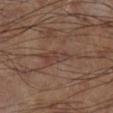Clinical impression: The lesion was photographed on a routine skin check and not biopsied; there is no pathology result. Image and clinical context: A region of skin cropped from a whole-body photographic capture, roughly 15 mm wide. About 3.5 mm across. Located on the right lower leg. An algorithmic analysis of the crop reported a border-irregularity index near 4/10 and a within-lesion color-variation index near 2.5/10. This is a cross-polarized tile.